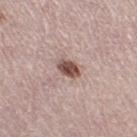Captured during whole-body skin photography for melanoma surveillance; the lesion was not biopsied.
The lesion's longest dimension is about 3 mm.
Captured under white-light illumination.
A region of skin cropped from a whole-body photographic capture, roughly 15 mm wide.
Located on the right thigh.
A female patient, aged 48 to 52.